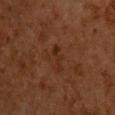<record>
<biopsy_status>not biopsied; imaged during a skin examination</biopsy_status>
<patient>
  <sex>male</sex>
  <age_approx>65</age_approx>
</patient>
<site>front of the torso</site>
<image>
  <source>total-body photography crop</source>
  <field_of_view_mm>15</field_of_view_mm>
</image>
</record>